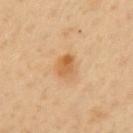<tbp_lesion>
<lesion_size>
  <long_diameter_mm_approx>2.5</long_diameter_mm_approx>
</lesion_size>
<image>
  <source>total-body photography crop</source>
  <field_of_view_mm>15</field_of_view_mm>
</image>
<site>left upper arm</site>
<patient>
  <sex>female</sex>
  <age_approx>50</age_approx>
</patient>
<lighting>cross-polarized</lighting>
<automated_metrics>
  <cielab_L>59</cielab_L>
  <cielab_a>22</cielab_a>
  <cielab_b>41</cielab_b>
  <vs_skin_contrast_norm>7.5</vs_skin_contrast_norm>
  <border_irregularity_0_10>2.0</border_irregularity_0_10>
  <color_variation_0_10>4.0</color_variation_0_10>
  <peripheral_color_asymmetry>1.5</peripheral_color_asymmetry>
</automated_metrics>
</tbp_lesion>